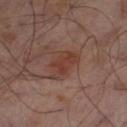| key | value |
|---|---|
| workup | catalogued during a skin exam; not biopsied |
| patient | male, aged 63–67 |
| image | 15 mm crop, total-body photography |
| lesion diameter | ~4.5 mm (longest diameter) |
| body site | the left thigh |